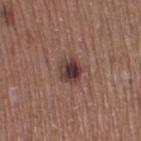A female patient approximately 55 years of age. The tile uses white-light illumination. Located on the right thigh. Measured at roughly 3 mm in maximum diameter. Cropped from a whole-body photographic skin survey; the tile spans about 15 mm.